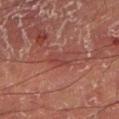Recorded during total-body skin imaging; not selected for excision or biopsy.
Captured under cross-polarized illumination.
Cropped from a whole-body photographic skin survey; the tile spans about 15 mm.
A male subject in their mid-50s.
Located on the left lower leg.